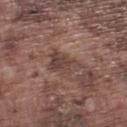The lesion was photographed on a routine skin check and not biopsied; there is no pathology result. From the left lower leg. A male patient, aged 73 to 77. A roughly 15 mm field-of-view crop from a total-body skin photograph. The lesion's longest dimension is about 4 mm. The tile uses white-light illumination. Automated image analysis of the tile measured an area of roughly 7.5 mm² and a symmetry-axis asymmetry near 0.4. The analysis additionally found a lesion color around L≈42 a*≈18 b*≈21 in CIELAB, about 8 CIELAB-L* units darker than the surrounding skin, and a normalized lesion–skin contrast near 7.